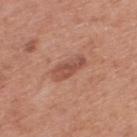Image and clinical context:
The subject is a male aged 53 to 57. Cropped from a whole-body photographic skin survey; the tile spans about 15 mm. The tile uses white-light illumination. On the upper back. Automated image analysis of the tile measured a lesion area of about 6 mm² and an eccentricity of roughly 0.95.